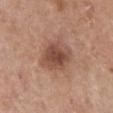Captured during whole-body skin photography for melanoma surveillance; the lesion was not biopsied.
On the chest.
An algorithmic analysis of the crop reported a lesion area of about 12 mm² and a symmetry-axis asymmetry near 0.2. It also reported a border-irregularity rating of about 2/10. And it measured an automated nevus-likeness rating near 50 out of 100 and a lesion-detection confidence of about 100/100.
Longest diameter approximately 4.5 mm.
Imaged with white-light lighting.
A female subject, aged approximately 65.
Cropped from a whole-body photographic skin survey; the tile spans about 15 mm.